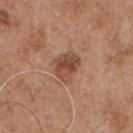The total-body-photography lesion software estimated a symmetry-axis asymmetry near 0.25. And it measured a nevus-likeness score of about 70/100. This is a white-light tile. A male patient aged around 55. The lesion is on the chest. A 15 mm crop from a total-body photograph taken for skin-cancer surveillance. Longest diameter approximately 3.5 mm.